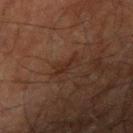follow-up: no biopsy performed (imaged during a skin exam) | patient: male, aged around 70 | acquisition: ~15 mm crop, total-body skin-cancer survey | location: the right upper arm.